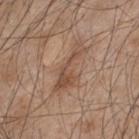This lesion was catalogued during total-body skin photography and was not selected for biopsy. A male patient aged 43–47. From the upper back. A 15 mm crop from a total-body photograph taken for skin-cancer surveillance.